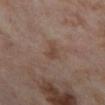Q: Was a biopsy performed?
A: no biopsy performed (imaged during a skin exam)
Q: How was the tile lit?
A: cross-polarized
Q: Where on the body is the lesion?
A: the left thigh
Q: How was this image acquired?
A: 15 mm crop, total-body photography
Q: Who is the patient?
A: female, in their mid-50s